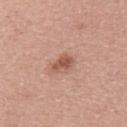| feature | finding |
|---|---|
| notes | no biopsy performed (imaged during a skin exam) |
| automated lesion analysis | a lesion color around L≈55 a*≈23 b*≈29 in CIELAB, roughly 11 lightness units darker than nearby skin, and a normalized lesion–skin contrast near 7.5; internal color variation of about 3 on a 0–10 scale and a peripheral color-asymmetry measure near 1; a classifier nevus-likeness of about 65/100 and a detector confidence of about 100 out of 100 that the crop contains a lesion |
| location | the left upper arm |
| imaging modality | ~15 mm tile from a whole-body skin photo |
| patient | female, aged approximately 55 |
| lighting | white-light illumination |
| lesion diameter | ~3 mm (longest diameter) |